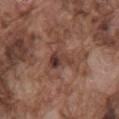<lesion>
  <biopsy_status>not biopsied; imaged during a skin examination</biopsy_status>
  <lighting>white-light</lighting>
  <site>back</site>
  <image>
    <source>total-body photography crop</source>
    <field_of_view_mm>15</field_of_view_mm>
  </image>
  <lesion_size>
    <long_diameter_mm_approx>3.5</long_diameter_mm_approx>
  </lesion_size>
  <patient>
    <sex>male</sex>
    <age_approx>75</age_approx>
  </patient>
</lesion>